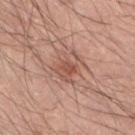Q: Was this lesion biopsied?
A: no biopsy performed (imaged during a skin exam)
Q: How large is the lesion?
A: ~3.5 mm (longest diameter)
Q: Lesion location?
A: the left lower leg
Q: Automated lesion metrics?
A: an area of roughly 8 mm² and a shape-asymmetry score of about 0.45 (0 = symmetric); a lesion–skin lightness drop of about 9 and a lesion-to-skin contrast of about 6 (normalized; higher = more distinct); border irregularity of about 5.5 on a 0–10 scale, a within-lesion color-variation index near 4.5/10, and radial color variation of about 1.5; a nevus-likeness score of about 0/100 and lesion-presence confidence of about 100/100
Q: What is the imaging modality?
A: ~15 mm crop, total-body skin-cancer survey
Q: How was the tile lit?
A: white-light illumination
Q: Patient demographics?
A: male, aged approximately 40